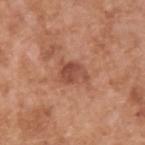Q: Is there a histopathology result?
A: catalogued during a skin exam; not biopsied
Q: Illumination type?
A: white-light
Q: What kind of image is this?
A: ~15 mm tile from a whole-body skin photo
Q: Lesion size?
A: ~3.5 mm (longest diameter)
Q: What are the patient's age and sex?
A: male, aged approximately 65
Q: Lesion location?
A: the upper back